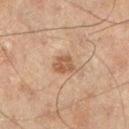Assessment:
The lesion was photographed on a routine skin check and not biopsied; there is no pathology result.
Image and clinical context:
Imaged with cross-polarized lighting. Cropped from a whole-body photographic skin survey; the tile spans about 15 mm. A male patient in their mid-40s. On the leg.